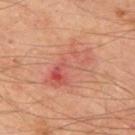<tbp_lesion>
<biopsy_status>not biopsied; imaged during a skin examination</biopsy_status>
<image>
  <source>total-body photography crop</source>
  <field_of_view_mm>15</field_of_view_mm>
</image>
<patient>
  <sex>male</sex>
  <age_approx>65</age_approx>
</patient>
<site>upper back</site>
<lesion_size>
  <long_diameter_mm_approx>6.5</long_diameter_mm_approx>
</lesion_size>
<lighting>cross-polarized</lighting>
</tbp_lesion>